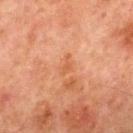| field | value |
|---|---|
| biopsy status | total-body-photography surveillance lesion; no biopsy |
| anatomic site | the chest |
| diameter | ≈2.5 mm |
| illumination | cross-polarized illumination |
| subject | male, aged 63–67 |
| image | ~15 mm tile from a whole-body skin photo |